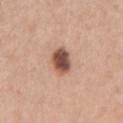biopsy status — imaged on a skin check; not biopsied
acquisition — ~15 mm crop, total-body skin-cancer survey
site — the right upper arm
image-analysis metrics — a mean CIELAB color near L≈51 a*≈21 b*≈27, about 18 CIELAB-L* units darker than the surrounding skin, and a normalized lesion–skin contrast near 12
lesion size — ≈3.5 mm
tile lighting — white-light
subject — female, aged 43 to 47